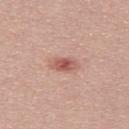Recorded during total-body skin imaging; not selected for excision or biopsy. Cropped from a total-body skin-imaging series; the visible field is about 15 mm. A male subject in their 30s.